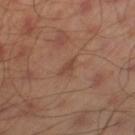Image and clinical context:
A close-up tile cropped from a whole-body skin photograph, about 15 mm across. The total-body-photography lesion software estimated a border-irregularity rating of about 3.5/10 and internal color variation of about 0 on a 0–10 scale. Measured at roughly 2.5 mm in maximum diameter. Located on the left thigh. A male patient in their mid-40s.